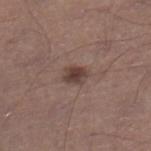The lesion was photographed on a routine skin check and not biopsied; there is no pathology result. The lesion is located on the right lower leg. A 15 mm close-up extracted from a 3D total-body photography capture. A male patient, about 45 years old. This is a white-light tile.